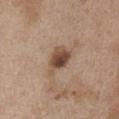Impression: The lesion was photographed on a routine skin check and not biopsied; there is no pathology result. Image and clinical context: A female patient, in their 40s. Measured at roughly 4.5 mm in maximum diameter. Captured under white-light illumination. On the left upper arm. The total-body-photography lesion software estimated an outline eccentricity of about 0.8 (0 = round, 1 = elongated) and a shape-asymmetry score of about 0.3 (0 = symmetric). The software also gave a classifier nevus-likeness of about 75/100 and a detector confidence of about 100 out of 100 that the crop contains a lesion. A 15 mm close-up tile from a total-body photography series done for melanoma screening.